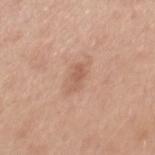No biopsy was performed on this lesion — it was imaged during a full skin examination and was not determined to be concerning.
A 15 mm close-up extracted from a 3D total-body photography capture.
The subject is a male roughly 30 years of age.
From the mid back.